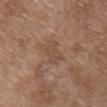This lesion was catalogued during total-body skin photography and was not selected for biopsy.
On the upper back.
The tile uses white-light illumination.
A female patient, approximately 70 years of age.
Automated tile analysis of the lesion measured a footprint of about 5.5 mm², a shape eccentricity near 0.75, and a shape-asymmetry score of about 0.25 (0 = symmetric). The software also gave a border-irregularity rating of about 3/10, a within-lesion color-variation index near 1.5/10, and radial color variation of about 0.5.
A lesion tile, about 15 mm wide, cut from a 3D total-body photograph.
Approximately 3 mm at its widest.